The subject is a male aged 58 to 62, a 15 mm crop from a total-body photograph taken for skin-cancer surveillance, the lesion's longest dimension is about 1.5 mm, the lesion is located on the back: 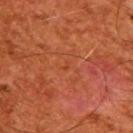Histopathologically confirmed as a superficial basal cell carcinoma.